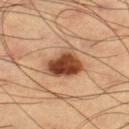Q: Was this lesion biopsied?
A: total-body-photography surveillance lesion; no biopsy
Q: What lighting was used for the tile?
A: cross-polarized
Q: Lesion location?
A: the right thigh
Q: How large is the lesion?
A: ≈4 mm
Q: What did automated image analysis measure?
A: a within-lesion color-variation index near 6.5/10 and radial color variation of about 2; an automated nevus-likeness rating near 100 out of 100
Q: Patient demographics?
A: male, aged 58 to 62
Q: What is the imaging modality?
A: total-body-photography crop, ~15 mm field of view